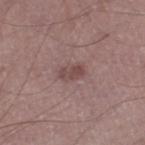A male subject, in their 60s. Imaged with white-light lighting. From the left thigh. Longest diameter approximately 2.5 mm. A region of skin cropped from a whole-body photographic capture, roughly 15 mm wide. An algorithmic analysis of the crop reported a mean CIELAB color near L≈45 a*≈19 b*≈19, roughly 9 lightness units darker than nearby skin, and a normalized lesion–skin contrast near 7. It also reported border irregularity of about 3 on a 0–10 scale, a color-variation rating of about 1/10, and peripheral color asymmetry of about 0. The analysis additionally found a classifier nevus-likeness of about 0/100 and lesion-presence confidence of about 100/100.